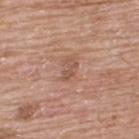- biopsy status — no biopsy performed (imaged during a skin exam)